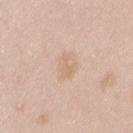biopsy status — imaged on a skin check; not biopsied
imaging modality — ~15 mm crop, total-body skin-cancer survey
subject — female, aged 23 to 27
tile lighting — white-light illumination
location — the left thigh